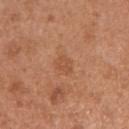notes: total-body-photography surveillance lesion; no biopsy.